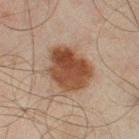This lesion was catalogued during total-body skin photography and was not selected for biopsy. A region of skin cropped from a whole-body photographic capture, roughly 15 mm wide. Imaged with cross-polarized lighting. The subject is a male aged approximately 45. Longest diameter approximately 5.5 mm. Automated image analysis of the tile measured a mean CIELAB color near L≈37 a*≈17 b*≈26 and a normalized lesion–skin contrast near 11. The analysis additionally found a border-irregularity index near 2/10, a color-variation rating of about 5/10, and a peripheral color-asymmetry measure near 2. The lesion is located on the left thigh.